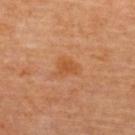{
  "biopsy_status": "not biopsied; imaged during a skin examination",
  "lesion_size": {
    "long_diameter_mm_approx": 2.5
  },
  "image": {
    "source": "total-body photography crop",
    "field_of_view_mm": 15
  },
  "patient": {
    "sex": "female",
    "age_approx": 65
  },
  "site": "back",
  "lighting": "cross-polarized"
}